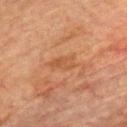No biopsy was performed on this lesion — it was imaged during a full skin examination and was not determined to be concerning.
The lesion is on the chest.
Cropped from a whole-body photographic skin survey; the tile spans about 15 mm.
A male subject in their mid-70s.
Captured under cross-polarized illumination.
Longest diameter approximately 3 mm.